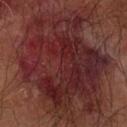location: the arm; diameter: ≈13.5 mm; image source: 15 mm crop, total-body photography; tile lighting: cross-polarized; patient: male, roughly 65 years of age.